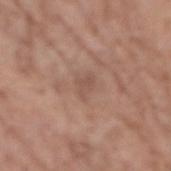A male patient about 70 years old.
Measured at roughly 2.5 mm in maximum diameter.
The lesion-visualizer software estimated a footprint of about 3.5 mm², an eccentricity of roughly 0.65, and a symmetry-axis asymmetry near 0.3. And it measured an average lesion color of about L≈52 a*≈19 b*≈26 (CIELAB) and a normalized lesion–skin contrast near 4.5. It also reported an automated nevus-likeness rating near 0 out of 100 and a detector confidence of about 100 out of 100 that the crop contains a lesion.
From the left forearm.
Captured under white-light illumination.
A region of skin cropped from a whole-body photographic capture, roughly 15 mm wide.